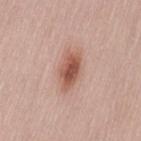| feature | finding |
|---|---|
| follow-up | no biopsy performed (imaged during a skin exam) |
| diameter | ≈4.5 mm |
| patient | female, aged around 55 |
| anatomic site | the lower back |
| lighting | white-light |
| image | 15 mm crop, total-body photography |